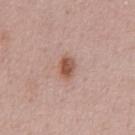The lesion was photographed on a routine skin check and not biopsied; there is no pathology result.